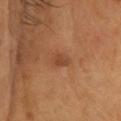The lesion was photographed on a routine skin check and not biopsied; there is no pathology result. The total-body-photography lesion software estimated an area of roughly 4 mm² and a shape-asymmetry score of about 0.25 (0 = symmetric). The analysis additionally found an average lesion color of about L≈46 a*≈23 b*≈34 (CIELAB), about 8 CIELAB-L* units darker than the surrounding skin, and a normalized lesion–skin contrast near 6. It also reported a border-irregularity index near 2/10 and internal color variation of about 1.5 on a 0–10 scale. Located on the head or neck. About 2.5 mm across. A female subject about 40 years old. Cropped from a whole-body photographic skin survey; the tile spans about 15 mm. The tile uses cross-polarized illumination.